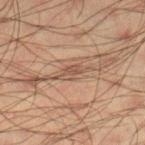Q: What did automated image analysis measure?
A: a lesion area of about 4.5 mm², a shape eccentricity near 0.9, and two-axis asymmetry of about 0.4; a mean CIELAB color near L≈48 a*≈17 b*≈27 and a normalized lesion–skin contrast near 6.5
Q: Lesion location?
A: the right lower leg
Q: Patient demographics?
A: male, approximately 35 years of age
Q: How was this image acquired?
A: ~15 mm crop, total-body skin-cancer survey
Q: How large is the lesion?
A: ~4 mm (longest diameter)